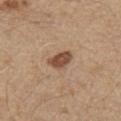The lesion was tiled from a total-body skin photograph and was not biopsied. From the chest. A male subject, aged 68–72. Automated tile analysis of the lesion measured a footprint of about 5 mm² and a shape-asymmetry score of about 0.3 (0 = symmetric). It also reported a border-irregularity rating of about 2.5/10 and radial color variation of about 1. A region of skin cropped from a whole-body photographic capture, roughly 15 mm wide.